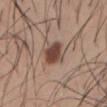Notes:
– lesion diameter · ~3 mm (longest diameter)
– imaging modality · total-body-photography crop, ~15 mm field of view
– image-analysis metrics · a lesion area of about 6.5 mm² and two-axis asymmetry of about 0.3; a within-lesion color-variation index near 3/10 and a peripheral color-asymmetry measure near 1
– subject · male, aged around 30
– body site · the front of the torso
– illumination · white-light illumination